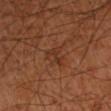Case summary:
– biopsy status · imaged on a skin check; not biopsied
– subject · male, aged around 60
– illumination · cross-polarized
– automated lesion analysis · an eccentricity of roughly 0.85 and a shape-asymmetry score of about 0.65 (0 = symmetric); a border-irregularity rating of about 7/10 and internal color variation of about 0 on a 0–10 scale
– anatomic site · the arm
– lesion diameter · about 3 mm
– image source · total-body-photography crop, ~15 mm field of view